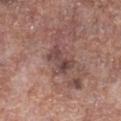follow-up — total-body-photography surveillance lesion; no biopsy | illumination — white-light illumination | subject — male, aged 53–57 | imaging modality — total-body-photography crop, ~15 mm field of view | body site — the right lower leg | size — ≈3.5 mm.